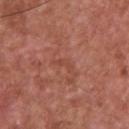Q: Was a biopsy performed?
A: imaged on a skin check; not biopsied